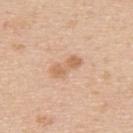Case summary:
– image — ~15 mm crop, total-body skin-cancer survey
– patient — male, in their mid- to late 30s
– site — the upper back